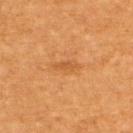Image and clinical context:
A roughly 15 mm field-of-view crop from a total-body skin photograph. Located on the upper back. The tile uses cross-polarized illumination. The patient is a male roughly 60 years of age. Approximately 3 mm at its widest.